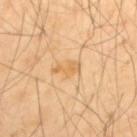The lesion was photographed on a routine skin check and not biopsied; there is no pathology result.
Imaged with cross-polarized lighting.
Approximately 3 mm at its widest.
A male subject approximately 40 years of age.
Cropped from a total-body skin-imaging series; the visible field is about 15 mm.
From the upper back.